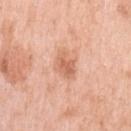Recorded during total-body skin imaging; not selected for excision or biopsy. Captured under white-light illumination. The total-body-photography lesion software estimated a footprint of about 6 mm², an outline eccentricity of about 0.75 (0 = round, 1 = elongated), and two-axis asymmetry of about 0.2. It also reported a mean CIELAB color near L≈64 a*≈25 b*≈34 and roughly 10 lightness units darker than nearby skin. The analysis additionally found a border-irregularity index near 2/10, a color-variation rating of about 3/10, and radial color variation of about 1. And it measured an automated nevus-likeness rating near 5 out of 100. Measured at roughly 3.5 mm in maximum diameter. Cropped from a whole-body photographic skin survey; the tile spans about 15 mm. The lesion is located on the left upper arm. The patient is a female in their 40s.